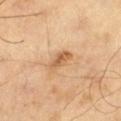Case summary:
– notes: total-body-photography surveillance lesion; no biopsy
– image: ~15 mm crop, total-body skin-cancer survey
– size: ~3 mm (longest diameter)
– tile lighting: cross-polarized
– subject: male, in their mid-60s